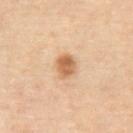Imaged during a routine full-body skin examination; the lesion was not biopsied and no histopathology is available. This is a cross-polarized tile. Located on the abdomen. A 15 mm close-up extracted from a 3D total-body photography capture. A male patient, aged approximately 70.